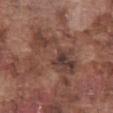notes: no biopsy performed (imaged during a skin exam)
imaging modality: total-body-photography crop, ~15 mm field of view
site: the front of the torso
subject: male, in their mid- to late 70s